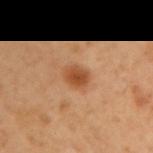Part of a total-body skin-imaging series; this lesion was reviewed on a skin check and was not flagged for biopsy. The subject is a male roughly 50 years of age. The lesion is on the right upper arm. An algorithmic analysis of the crop reported a color-variation rating of about 3/10 and peripheral color asymmetry of about 1. It also reported a classifier nevus-likeness of about 95/100 and a lesion-detection confidence of about 100/100. A 15 mm close-up tile from a total-body photography series done for melanoma screening. Captured under cross-polarized illumination.